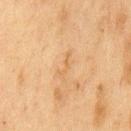<tbp_lesion>
  <site>front of the torso</site>
  <lighting>cross-polarized</lighting>
  <lesion_size>
    <long_diameter_mm_approx>3.0</long_diameter_mm_approx>
  </lesion_size>
  <patient>
    <sex>male</sex>
    <age_approx>75</age_approx>
  </patient>
  <image>
    <source>total-body photography crop</source>
    <field_of_view_mm>15</field_of_view_mm>
  </image>
</tbp_lesion>